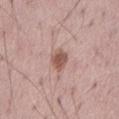Case summary:
* workup · imaged on a skin check; not biopsied
* lighting · white-light
* image · 15 mm crop, total-body photography
* subject · male, about 70 years old
* location · the mid back
* size · about 3 mm